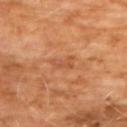No biopsy was performed on this lesion — it was imaged during a full skin examination and was not determined to be concerning. The patient is a male about 60 years old. Imaged with cross-polarized lighting. Located on the chest. About 3 mm across. A lesion tile, about 15 mm wide, cut from a 3D total-body photograph.